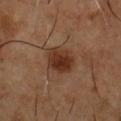Part of a total-body skin-imaging series; this lesion was reviewed on a skin check and was not flagged for biopsy.
Cropped from a whole-body photographic skin survey; the tile spans about 15 mm.
The patient is a male aged 53 to 57.
The tile uses cross-polarized illumination.
Approximately 3.5 mm at its widest.
From the chest.
The lesion-visualizer software estimated border irregularity of about 1.5 on a 0–10 scale, internal color variation of about 4.5 on a 0–10 scale, and radial color variation of about 1.5.